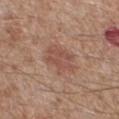workup: total-body-photography surveillance lesion; no biopsy
acquisition: ~15 mm tile from a whole-body skin photo
automated metrics: a lesion color around L≈51 a*≈22 b*≈26 in CIELAB and a lesion–skin lightness drop of about 8; a border-irregularity index near 3/10
site: the right lower leg
patient: male, approximately 65 years of age
lesion diameter: ≈4.5 mm